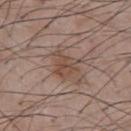- notes · catalogued during a skin exam; not biopsied
- diameter · about 3.5 mm
- imaging modality · 15 mm crop, total-body photography
- lighting · white-light illumination
- subject · male, aged approximately 55
- body site · the front of the torso